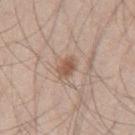Notes:
• biopsy status: catalogued during a skin exam; not biopsied
• image: ~15 mm crop, total-body skin-cancer survey
• site: the right thigh
• subject: male, aged 43–47
• size: ≈3 mm
• illumination: white-light illumination